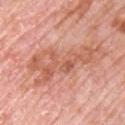<lesion>
  <biopsy_status>not biopsied; imaged during a skin examination</biopsy_status>
  <lesion_size>
    <long_diameter_mm_approx>10.0</long_diameter_mm_approx>
  </lesion_size>
  <patient>
    <sex>male</sex>
    <age_approx>80</age_approx>
  </patient>
  <image>
    <source>total-body photography crop</source>
    <field_of_view_mm>15</field_of_view_mm>
  </image>
  <site>chest</site>
</lesion>